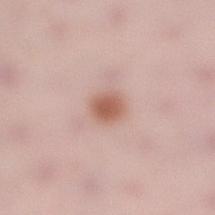Impression: Imaged during a routine full-body skin examination; the lesion was not biopsied and no histopathology is available. Context: A 15 mm close-up tile from a total-body photography series done for melanoma screening. Located on the right lower leg. A female subject in their 30s. Imaged with white-light lighting. Longest diameter approximately 2.5 mm.